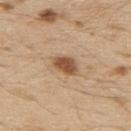Q: Was this lesion biopsied?
A: no biopsy performed (imaged during a skin exam)
Q: How was the tile lit?
A: white-light
Q: What did automated image analysis measure?
A: an automated nevus-likeness rating near 95 out of 100 and a lesion-detection confidence of about 100/100
Q: How large is the lesion?
A: ≈3.5 mm
Q: What kind of image is this?
A: ~15 mm tile from a whole-body skin photo
Q: Lesion location?
A: the back
Q: Patient demographics?
A: male, in their 70s